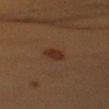Assessment:
Imaged during a routine full-body skin examination; the lesion was not biopsied and no histopathology is available.
Background:
This image is a 15 mm lesion crop taken from a total-body photograph. A male subject about 40 years old. Longest diameter approximately 3 mm. Located on the arm.